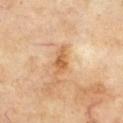Context: A region of skin cropped from a whole-body photographic capture, roughly 15 mm wide. From the chest. A male patient roughly 70 years of age. Captured under cross-polarized illumination. The recorded lesion diameter is about 3.5 mm.